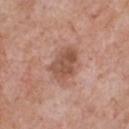– biopsy status — catalogued during a skin exam; not biopsied
– acquisition — total-body-photography crop, ~15 mm field of view
– lesion size — about 4.5 mm
– anatomic site — the chest
– subject — male, roughly 60 years of age
– image-analysis metrics — a lesion area of about 10 mm², an outline eccentricity of about 0.75 (0 = round, 1 = elongated), and a shape-asymmetry score of about 0.25 (0 = symmetric); a lesion–skin lightness drop of about 11 and a lesion-to-skin contrast of about 7.5 (normalized; higher = more distinct); an automated nevus-likeness rating near 10 out of 100 and lesion-presence confidence of about 100/100
– illumination — white-light illumination